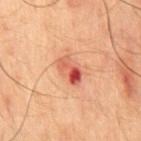• notes — no biopsy performed (imaged during a skin exam)
• lighting — cross-polarized illumination
• site — the chest
• subject — male, aged 48–52
• image — ~15 mm tile from a whole-body skin photo